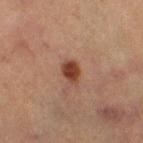Q: Was this lesion biopsied?
A: total-body-photography surveillance lesion; no biopsy
Q: Patient demographics?
A: female, about 60 years old
Q: Where on the body is the lesion?
A: the right thigh
Q: How was this image acquired?
A: 15 mm crop, total-body photography